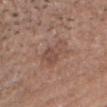| field | value |
|---|---|
| biopsy status | catalogued during a skin exam; not biopsied |
| anatomic site | the head or neck |
| image | ~15 mm tile from a whole-body skin photo |
| size | ≈4.5 mm |
| subject | male, aged 48–52 |
| lighting | white-light |